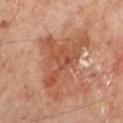biopsy status: imaged on a skin check; not biopsied
anatomic site: the left lower leg
subject: male, aged approximately 50
lighting: cross-polarized
size: about 8.5 mm
image source: ~15 mm tile from a whole-body skin photo